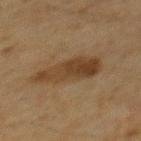<lesion>
  <biopsy_status>not biopsied; imaged during a skin examination</biopsy_status>
  <patient>
    <sex>male</sex>
    <age_approx>60</age_approx>
  </patient>
  <image>
    <source>total-body photography crop</source>
    <field_of_view_mm>15</field_of_view_mm>
  </image>
  <site>mid back</site>
  <automated_metrics>
    <eccentricity>0.9</eccentricity>
    <cielab_L>33</cielab_L>
    <cielab_a>14</cielab_a>
    <cielab_b>27</cielab_b>
    <vs_skin_darker_L>8.0</vs_skin_darker_L>
    <vs_skin_contrast_norm>8.5</vs_skin_contrast_norm>
  </automated_metrics>
  <lighting>cross-polarized</lighting>
</lesion>